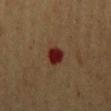No biopsy was performed on this lesion — it was imaged during a full skin examination and was not determined to be concerning. A male subject, approximately 60 years of age. An algorithmic analysis of the crop reported a footprint of about 4.5 mm², a shape eccentricity near 0.7, and a shape-asymmetry score of about 0.25 (0 = symmetric). And it measured a mean CIELAB color near L≈22 a*≈26 b*≈23, about 13 CIELAB-L* units darker than the surrounding skin, and a normalized lesion–skin contrast near 14.5. The analysis additionally found a border-irregularity rating of about 2/10 and peripheral color asymmetry of about 0.5. It also reported a classifier nevus-likeness of about 0/100 and a lesion-detection confidence of about 100/100. A roughly 15 mm field-of-view crop from a total-body skin photograph. From the left upper arm. About 3 mm across.